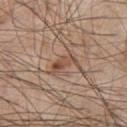– follow-up · catalogued during a skin exam; not biopsied
– image source · 15 mm crop, total-body photography
– image-analysis metrics · an average lesion color of about L≈49 a*≈19 b*≈29 (CIELAB), roughly 9 lightness units darker than nearby skin, and a lesion-to-skin contrast of about 7 (normalized; higher = more distinct); internal color variation of about 1.5 on a 0–10 scale and a peripheral color-asymmetry measure near 0.5
– lesion size · ≈3 mm
– patient · male, approximately 60 years of age
– anatomic site · the chest